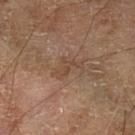workup: no biopsy performed (imaged during a skin exam)
image-analysis metrics: a lesion area of about 4 mm² and two-axis asymmetry of about 0.7; a normalized border contrast of about 5.5
subject: male, roughly 70 years of age
size: ≈3.5 mm
image: 15 mm crop, total-body photography
body site: the left lower leg